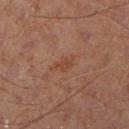workup: imaged on a skin check; not biopsied
body site: the left leg
subject: male, approximately 60 years of age
acquisition: ~15 mm tile from a whole-body skin photo